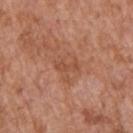Imaged during a routine full-body skin examination; the lesion was not biopsied and no histopathology is available. On the upper back. The subject is a male in their mid-60s. A region of skin cropped from a whole-body photographic capture, roughly 15 mm wide. The tile uses white-light illumination. The recorded lesion diameter is about 3 mm.